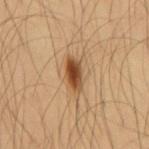No biopsy was performed on this lesion — it was imaged during a full skin examination and was not determined to be concerning.